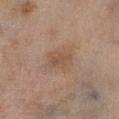<case>
<biopsy_status>not biopsied; imaged during a skin examination</biopsy_status>
<lesion_size>
  <long_diameter_mm_approx>3.0</long_diameter_mm_approx>
</lesion_size>
<patient>
  <sex>female</sex>
  <age_approx>60</age_approx>
</patient>
<lighting>cross-polarized</lighting>
<site>leg</site>
<automated_metrics>
  <border_irregularity_0_10>2.5</border_irregularity_0_10>
  <color_variation_0_10>2.5</color_variation_0_10>
  <peripheral_color_asymmetry>1.0</peripheral_color_asymmetry>
</automated_metrics>
<image>
  <source>total-body photography crop</source>
  <field_of_view_mm>15</field_of_view_mm>
</image>
</case>